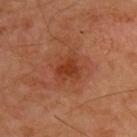follow-up: total-body-photography surveillance lesion; no biopsy
location: the back
lesion size: ~4 mm (longest diameter)
subject: male, roughly 50 years of age
acquisition: total-body-photography crop, ~15 mm field of view
illumination: cross-polarized illumination